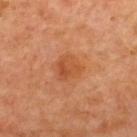Q: Is there a histopathology result?
A: no biopsy performed (imaged during a skin exam)
Q: Who is the patient?
A: aged 63–67
Q: How large is the lesion?
A: about 3.5 mm
Q: What is the anatomic site?
A: the upper back
Q: What kind of image is this?
A: 15 mm crop, total-body photography